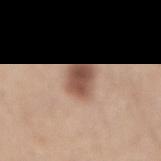follow-up — imaged on a skin check; not biopsied
site — the mid back
subject — male, approximately 50 years of age
image source — total-body-photography crop, ~15 mm field of view
TBP lesion metrics — an eccentricity of roughly 0.65 and a shape-asymmetry score of about 0.25 (0 = symmetric); a border-irregularity rating of about 2.5/10, a color-variation rating of about 4.5/10, and peripheral color asymmetry of about 1.5; an automated nevus-likeness rating near 100 out of 100
lighting — white-light illumination